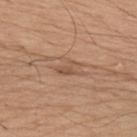Clinical impression:
The lesion was tiled from a total-body skin photograph and was not biopsied.
Image and clinical context:
The lesion is located on the upper back. This is a white-light tile. A 15 mm close-up tile from a total-body photography series done for melanoma screening. A male patient roughly 65 years of age. The total-body-photography lesion software estimated a lesion area of about 3.5 mm², a shape eccentricity near 0.85, and two-axis asymmetry of about 0.35. It also reported a border-irregularity rating of about 4/10, a color-variation rating of about 2/10, and radial color variation of about 0.5. And it measured a nevus-likeness score of about 10/100 and lesion-presence confidence of about 90/100.